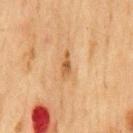Imaged during a routine full-body skin examination; the lesion was not biopsied and no histopathology is available.
The lesion's longest dimension is about 3 mm.
A region of skin cropped from a whole-body photographic capture, roughly 15 mm wide.
An algorithmic analysis of the crop reported an eccentricity of roughly 0.9 and a shape-asymmetry score of about 0.3 (0 = symmetric). It also reported a mean CIELAB color near L≈47 a*≈18 b*≈35, roughly 9 lightness units darker than nearby skin, and a normalized lesion–skin contrast near 7.5.
The lesion is located on the chest.
A male patient, about 75 years old.